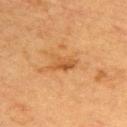follow-up = imaged on a skin check; not biopsied
subject = female, approximately 55 years of age
image = total-body-photography crop, ~15 mm field of view
site = the back